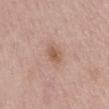{
  "biopsy_status": "not biopsied; imaged during a skin examination",
  "lighting": "white-light",
  "lesion_size": {
    "long_diameter_mm_approx": 3.0
  },
  "site": "abdomen",
  "image": {
    "source": "total-body photography crop",
    "field_of_view_mm": 15
  },
  "automated_metrics": {
    "area_mm2_approx": 3.5,
    "eccentricity": 0.8,
    "shape_asymmetry": 0.15,
    "nevus_likeness_0_100": 50,
    "lesion_detection_confidence_0_100": 100
  },
  "patient": {
    "sex": "male",
    "age_approx": 70
  }
}